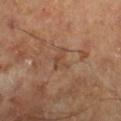{
  "biopsy_status": "not biopsied; imaged during a skin examination",
  "lesion_size": {
    "long_diameter_mm_approx": 2.5
  },
  "lighting": "cross-polarized",
  "patient": {
    "sex": "male",
    "age_approx": 65
  },
  "image": {
    "source": "total-body photography crop",
    "field_of_view_mm": 15
  }
}